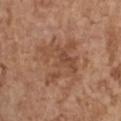Background:
From the chest. Imaged with white-light lighting. Cropped from a whole-body photographic skin survey; the tile spans about 15 mm. The recorded lesion diameter is about 5 mm. A female subject, aged approximately 65.